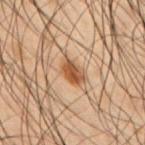This lesion was catalogued during total-body skin photography and was not selected for biopsy. Approximately 3 mm at its widest. A male subject aged 48 to 52. Automated tile analysis of the lesion measured a footprint of about 4.5 mm², an eccentricity of roughly 0.75, and two-axis asymmetry of about 0.25. The software also gave a mean CIELAB color near L≈39 a*≈18 b*≈29, about 11 CIELAB-L* units darker than the surrounding skin, and a lesion-to-skin contrast of about 10 (normalized; higher = more distinct). The analysis additionally found a color-variation rating of about 3/10 and peripheral color asymmetry of about 1. The software also gave lesion-presence confidence of about 100/100. Located on the right upper arm. Cropped from a whole-body photographic skin survey; the tile spans about 15 mm. The tile uses cross-polarized illumination.